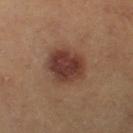Assessment: This lesion was catalogued during total-body skin photography and was not selected for biopsy. Image and clinical context: Captured under cross-polarized illumination. Longest diameter approximately 5.5 mm. The subject is a male aged 63 to 67. A lesion tile, about 15 mm wide, cut from a 3D total-body photograph. The lesion is located on the leg.